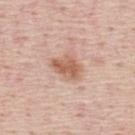The lesion was tiled from a total-body skin photograph and was not biopsied. Located on the mid back. This is a white-light tile. A male subject, roughly 45 years of age. This image is a 15 mm lesion crop taken from a total-body photograph.